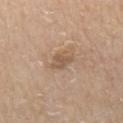- follow-up: catalogued during a skin exam; not biopsied
- image: ~15 mm tile from a whole-body skin photo
- patient: male, aged 78 to 82
- tile lighting: white-light illumination
- body site: the right upper arm